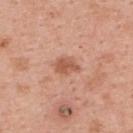<case>
<lesion_size>
  <long_diameter_mm_approx>3.0</long_diameter_mm_approx>
</lesion_size>
<lighting>white-light</lighting>
<site>upper back</site>
<image>
  <source>total-body photography crop</source>
  <field_of_view_mm>15</field_of_view_mm>
</image>
<patient>
  <sex>female</sex>
  <age_approx>50</age_approx>
</patient>
</case>